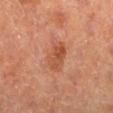Captured during whole-body skin photography for melanoma surveillance; the lesion was not biopsied. A 15 mm crop from a total-body photograph taken for skin-cancer surveillance. A male subject aged approximately 65. Captured under cross-polarized illumination. On the left lower leg.